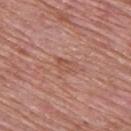Q: Was this lesion biopsied?
A: imaged on a skin check; not biopsied
Q: What did automated image analysis measure?
A: an outline eccentricity of about 0.8 (0 = round, 1 = elongated) and a shape-asymmetry score of about 0.4 (0 = symmetric); a lesion color around L≈53 a*≈23 b*≈29 in CIELAB, a lesion–skin lightness drop of about 6, and a normalized border contrast of about 5
Q: What is the lesion's diameter?
A: ~2.5 mm (longest diameter)
Q: What are the patient's age and sex?
A: male, approximately 50 years of age
Q: Where on the body is the lesion?
A: the upper back
Q: What kind of image is this?
A: ~15 mm tile from a whole-body skin photo
Q: What lighting was used for the tile?
A: white-light illumination